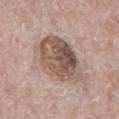| feature | finding |
|---|---|
| notes | imaged on a skin check; not biopsied |
| patient | male, aged approximately 70 |
| illumination | white-light |
| body site | the abdomen |
| image source | 15 mm crop, total-body photography |
| diameter | ≈7 mm |